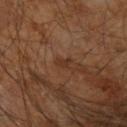Assessment: The lesion was photographed on a routine skin check and not biopsied; there is no pathology result. Context: On the right forearm. Measured at roughly 2 mm in maximum diameter. A male subject aged around 60. A roughly 15 mm field-of-view crop from a total-body skin photograph. Automated image analysis of the tile measured an outline eccentricity of about 0.8 (0 = round, 1 = elongated) and a shape-asymmetry score of about 0.4 (0 = symmetric). And it measured an average lesion color of about L≈29 a*≈18 b*≈26 (CIELAB). It also reported a nevus-likeness score of about 0/100 and a lesion-detection confidence of about 100/100.